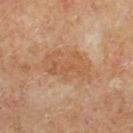The lesion is on the right lower leg.
A roughly 15 mm field-of-view crop from a total-body skin photograph.
A male subject, aged 63 to 67.